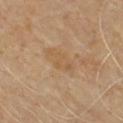This lesion was catalogued during total-body skin photography and was not selected for biopsy.
A male patient, aged around 60.
A 15 mm crop from a total-body photograph taken for skin-cancer surveillance.
About 4 mm across.
The tile uses cross-polarized illumination.
On the chest.
Automated tile analysis of the lesion measured an area of roughly 5 mm² and an eccentricity of roughly 0.9.